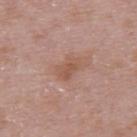Imaged during a routine full-body skin examination; the lesion was not biopsied and no histopathology is available.
A close-up tile cropped from a whole-body skin photograph, about 15 mm across.
The lesion is on the back.
A female patient, aged 28 to 32.